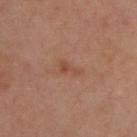Notes:
• TBP lesion metrics: a lesion area of about 2.5 mm², a shape eccentricity near 0.9, and a shape-asymmetry score of about 0.55 (0 = symmetric); an average lesion color of about L≈45 a*≈21 b*≈30 (CIELAB), a lesion–skin lightness drop of about 6, and a normalized lesion–skin contrast near 5.5; internal color variation of about 0.5 on a 0–10 scale and radial color variation of about 0
• acquisition: ~15 mm crop, total-body skin-cancer survey
• subject: male, in their mid-30s
• location: the upper back